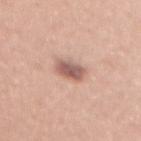The lesion was tiled from a total-body skin photograph and was not biopsied.